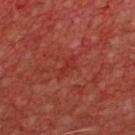Q: Patient demographics?
A: male, in their 60s
Q: How was this image acquired?
A: ~15 mm tile from a whole-body skin photo
Q: Automated lesion metrics?
A: a lesion color around L≈33 a*≈34 b*≈29 in CIELAB, about 5 CIELAB-L* units darker than the surrounding skin, and a normalized border contrast of about 4.5; a border-irregularity index near 4/10 and a within-lesion color-variation index near 0.5/10
Q: Where on the body is the lesion?
A: the chest
Q: What lighting was used for the tile?
A: cross-polarized